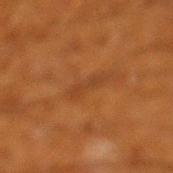Recorded during total-body skin imaging; not selected for excision or biopsy. Cropped from a total-body skin-imaging series; the visible field is about 15 mm. The lesion is located on the left lower leg. Imaged with cross-polarized lighting. A male patient aged 58–62. The lesion's longest dimension is about 3 mm.